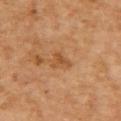A female patient, roughly 60 years of age. The lesion is on the upper back. Approximately 3 mm at its widest. A roughly 15 mm field-of-view crop from a total-body skin photograph.